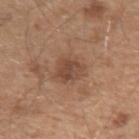No biopsy was performed on this lesion — it was imaged during a full skin examination and was not determined to be concerning.
A region of skin cropped from a whole-body photographic capture, roughly 15 mm wide.
From the left upper arm.
The lesion's longest dimension is about 3.5 mm.
Imaged with white-light lighting.
The patient is a male aged 48–52.